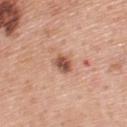Case summary:
• biopsy status: imaged on a skin check; not biopsied
• patient: male, aged 58 to 62
• lesion size: about 2.5 mm
• lighting: white-light illumination
• acquisition: ~15 mm crop, total-body skin-cancer survey
• body site: the upper back